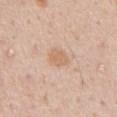Notes:
• workup: total-body-photography surveillance lesion; no biopsy
• location: the front of the torso
• patient: male, aged approximately 55
• diameter: about 3 mm
• image: total-body-photography crop, ~15 mm field of view
• illumination: white-light